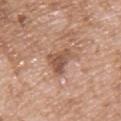{
  "biopsy_status": "not biopsied; imaged during a skin examination",
  "image": {
    "source": "total-body photography crop",
    "field_of_view_mm": 15
  },
  "lighting": "white-light",
  "patient": {
    "sex": "male",
    "age_approx": 50
  },
  "site": "upper back",
  "automated_metrics": {
    "area_mm2_approx": 7.5,
    "eccentricity": 0.8,
    "shape_asymmetry": 0.5,
    "cielab_L": 53,
    "cielab_a": 20,
    "cielab_b": 29,
    "vs_skin_darker_L": 11.0,
    "nevus_likeness_0_100": 0,
    "lesion_detection_confidence_0_100": 100
  },
  "lesion_size": {
    "long_diameter_mm_approx": 4.0
  }
}